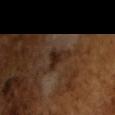Assessment: Recorded during total-body skin imaging; not selected for excision or biopsy. Image and clinical context: An algorithmic analysis of the crop reported an average lesion color of about L≈24 a*≈15 b*≈23 (CIELAB), about 8 CIELAB-L* units darker than the surrounding skin, and a normalized border contrast of about 9. It also reported a border-irregularity rating of about 5.5/10, a color-variation rating of about 2.5/10, and a peripheral color-asymmetry measure near 1. It also reported an automated nevus-likeness rating near 0 out of 100 and a detector confidence of about 95 out of 100 that the crop contains a lesion. Cropped from a whole-body photographic skin survey; the tile spans about 15 mm. A male subject, about 65 years old. Approximately 3.5 mm at its widest.